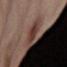Part of a total-body skin-imaging series; this lesion was reviewed on a skin check and was not flagged for biopsy. This image is a 15 mm lesion crop taken from a total-body photograph. A female subject aged approximately 55. The lesion is located on the abdomen.